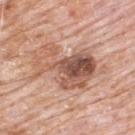  site: upper back
  image:
    source: total-body photography crop
    field_of_view_mm: 15
  patient:
    sex: male
    age_approx: 80